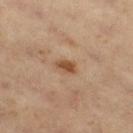| feature | finding |
|---|---|
| image | 15 mm crop, total-body photography |
| subject | male, aged 58–62 |
| automated lesion analysis | an average lesion color of about L≈46 a*≈19 b*≈32 (CIELAB), roughly 10 lightness units darker than nearby skin, and a normalized lesion–skin contrast near 8.5; an automated nevus-likeness rating near 85 out of 100 |
| lesion diameter | ~2.5 mm (longest diameter) |
| location | the right thigh |